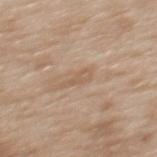Q: Is there a histopathology result?
A: no biopsy performed (imaged during a skin exam)
Q: How large is the lesion?
A: ≈3 mm
Q: What is the imaging modality?
A: ~15 mm crop, total-body skin-cancer survey
Q: Who is the patient?
A: female, roughly 40 years of age
Q: What did automated image analysis measure?
A: an average lesion color of about L≈58 a*≈16 b*≈30 (CIELAB), about 7 CIELAB-L* units darker than the surrounding skin, and a lesion-to-skin contrast of about 5 (normalized; higher = more distinct)
Q: Lesion location?
A: the mid back